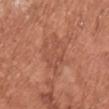Findings:
• notes: imaged on a skin check; not biopsied
• size: ≈5.5 mm
• site: the back
• acquisition: ~15 mm tile from a whole-body skin photo
• subject: female, aged 73–77
• illumination: white-light illumination
• image-analysis metrics: a footprint of about 12 mm², a shape eccentricity near 0.7, and a symmetry-axis asymmetry near 0.3; an average lesion color of about L≈51 a*≈25 b*≈31 (CIELAB), a lesion–skin lightness drop of about 6, and a normalized border contrast of about 4.5; a nevus-likeness score of about 0/100 and a detector confidence of about 100 out of 100 that the crop contains a lesion